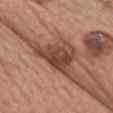Assessment: The lesion was photographed on a routine skin check and not biopsied; there is no pathology result. Background: A 15 mm close-up tile from a total-body photography series done for melanoma screening. The subject is a female aged 58–62. Approximately 7.5 mm at its widest. An algorithmic analysis of the crop reported an area of roughly 16 mm², an outline eccentricity of about 0.75 (0 = round, 1 = elongated), and a shape-asymmetry score of about 0.55 (0 = symmetric). And it measured a lesion color around L≈44 a*≈22 b*≈26 in CIELAB, roughly 13 lightness units darker than nearby skin, and a normalized border contrast of about 9.5. It also reported a border-irregularity index near 6.5/10, a color-variation rating of about 5/10, and radial color variation of about 1.5. The software also gave a classifier nevus-likeness of about 0/100 and a detector confidence of about 80 out of 100 that the crop contains a lesion. Located on the front of the torso.